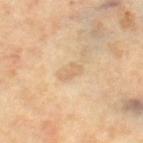| feature | finding |
|---|---|
| biopsy status | imaged on a skin check; not biopsied |
| patient | female, about 65 years old |
| image source | ~15 mm tile from a whole-body skin photo |
| size | about 2.5 mm |
| location | the right thigh |
| TBP lesion metrics | about 7 CIELAB-L* units darker than the surrounding skin; an automated nevus-likeness rating near 0 out of 100 |